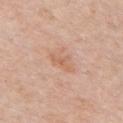Case summary:
– biopsy status: imaged on a skin check; not biopsied
– patient: female, in their 70s
– imaging modality: ~15 mm tile from a whole-body skin photo
– anatomic site: the chest